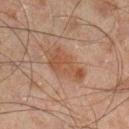Q: Is there a histopathology result?
A: total-body-photography surveillance lesion; no biopsy
Q: Automated lesion metrics?
A: border irregularity of about 3.5 on a 0–10 scale and a color-variation rating of about 3/10
Q: What is the anatomic site?
A: the right lower leg
Q: What kind of image is this?
A: 15 mm crop, total-body photography
Q: How was the tile lit?
A: cross-polarized illumination
Q: Lesion size?
A: about 5 mm
Q: What are the patient's age and sex?
A: male, approximately 45 years of age